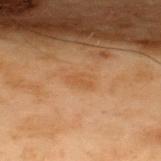Clinical impression: The lesion was tiled from a total-body skin photograph and was not biopsied. Clinical summary: Approximately 2.5 mm at its widest. Cropped from a total-body skin-imaging series; the visible field is about 15 mm. The total-body-photography lesion software estimated a mean CIELAB color near L≈43 a*≈19 b*≈33, a lesion–skin lightness drop of about 5, and a lesion-to-skin contrast of about 4.5 (normalized; higher = more distinct). And it measured border irregularity of about 3 on a 0–10 scale and a color-variation rating of about 1/10. The software also gave a nevus-likeness score of about 0/100 and lesion-presence confidence of about 100/100. A male patient in their mid-50s. The lesion is located on the upper back.